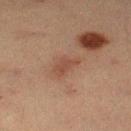Notes:
– biopsy status: total-body-photography surveillance lesion; no biopsy
– image: 15 mm crop, total-body photography
– patient: female, about 55 years old
– site: the left lower leg